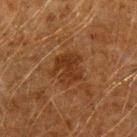Recorded during total-body skin imaging; not selected for excision or biopsy.
A male subject, aged 58 to 62.
Automated image analysis of the tile measured a footprint of about 10 mm² and two-axis asymmetry of about 0.25. The analysis additionally found internal color variation of about 2 on a 0–10 scale and radial color variation of about 1.
Measured at roughly 3.5 mm in maximum diameter.
A 15 mm close-up tile from a total-body photography series done for melanoma screening.
On the arm.
This is a cross-polarized tile.